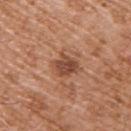Clinical impression:
No biopsy was performed on this lesion — it was imaged during a full skin examination and was not determined to be concerning.
Background:
From the upper back. A 15 mm close-up tile from a total-body photography series done for melanoma screening. The recorded lesion diameter is about 3.5 mm. The tile uses white-light illumination. The subject is a male in their mid- to late 70s.